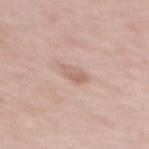Context:
An algorithmic analysis of the crop reported about 9 CIELAB-L* units darker than the surrounding skin and a lesion-to-skin contrast of about 6 (normalized; higher = more distinct). Cropped from a whole-body photographic skin survey; the tile spans about 15 mm. From the back. About 2.5 mm across. The tile uses white-light illumination. A male patient, approximately 55 years of age.